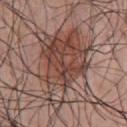workup — imaged on a skin check; not biopsied | image — ~15 mm crop, total-body skin-cancer survey | patient — male, approximately 55 years of age | body site — the front of the torso | tile lighting — white-light illumination | diameter — about 10.5 mm.